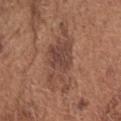Clinical impression:
Captured during whole-body skin photography for melanoma surveillance; the lesion was not biopsied.
Acquisition and patient details:
Captured under white-light illumination. A region of skin cropped from a whole-body photographic capture, roughly 15 mm wide. The lesion's longest dimension is about 9 mm. Located on the head or neck. The subject is a male aged 73 to 77. Automated tile analysis of the lesion measured an average lesion color of about L≈46 a*≈20 b*≈26 (CIELAB), a lesion–skin lightness drop of about 8, and a normalized lesion–skin contrast near 6.5.